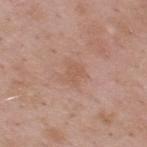<case>
<biopsy_status>not biopsied; imaged during a skin examination</biopsy_status>
<site>back</site>
<image>
  <source>total-body photography crop</source>
  <field_of_view_mm>15</field_of_view_mm>
</image>
<patient>
  <sex>male</sex>
  <age_approx>55</age_approx>
</patient>
<lesion_size>
  <long_diameter_mm_approx>2.5</long_diameter_mm_approx>
</lesion_size>
</case>